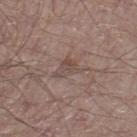The lesion was photographed on a routine skin check and not biopsied; there is no pathology result.
A 15 mm close-up extracted from a 3D total-body photography capture.
Imaged with white-light lighting.
A male patient, in their mid-50s.
Automated tile analysis of the lesion measured an area of roughly 4 mm² and a shape eccentricity near 0.75. It also reported a lesion color around L≈47 a*≈15 b*≈21 in CIELAB, a lesion–skin lightness drop of about 7, and a lesion-to-skin contrast of about 5.5 (normalized; higher = more distinct). And it measured a border-irregularity index near 5.5/10, internal color variation of about 2.5 on a 0–10 scale, and radial color variation of about 0.5. The software also gave an automated nevus-likeness rating near 0 out of 100 and a lesion-detection confidence of about 95/100.
The lesion is located on the leg.